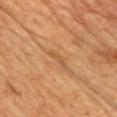Recorded during total-body skin imaging; not selected for excision or biopsy. The subject is a male in their mid-60s. From the chest. The recorded lesion diameter is about 3 mm. Cropped from a whole-body photographic skin survey; the tile spans about 15 mm.